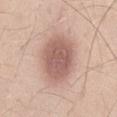The lesion was tiled from a total-body skin photograph and was not biopsied. A roughly 15 mm field-of-view crop from a total-body skin photograph. Located on the right thigh. The patient is a male aged 43–47. Imaged with white-light lighting.